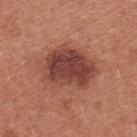Captured during whole-body skin photography for melanoma surveillance; the lesion was not biopsied.
Imaged with white-light lighting.
The patient is a female aged 33 to 37.
A region of skin cropped from a whole-body photographic capture, roughly 15 mm wide.
Automated image analysis of the tile measured an average lesion color of about L≈43 a*≈25 b*≈27 (CIELAB), about 13 CIELAB-L* units darker than the surrounding skin, and a lesion-to-skin contrast of about 10 (normalized; higher = more distinct). It also reported a detector confidence of about 100 out of 100 that the crop contains a lesion.
From the chest.